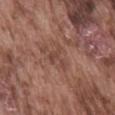Findings:
– notes — total-body-photography surveillance lesion; no biopsy
– body site — the mid back
– size — ≈3 mm
– acquisition — total-body-photography crop, ~15 mm field of view
– lighting — white-light illumination
– image-analysis metrics — a lesion area of about 4.5 mm², an outline eccentricity of about 0.6 (0 = round, 1 = elongated), and a symmetry-axis asymmetry near 0.65; a lesion color around L≈45 a*≈21 b*≈25 in CIELAB and a lesion-to-skin contrast of about 5 (normalized; higher = more distinct); border irregularity of about 8 on a 0–10 scale, internal color variation of about 1 on a 0–10 scale, and radial color variation of about 0
– patient — male, about 75 years old